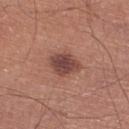| field | value |
|---|---|
| biopsy status | no biopsy performed (imaged during a skin exam) |
| image source | total-body-photography crop, ~15 mm field of view |
| lesion diameter | ~4 mm (longest diameter) |
| subject | male, aged 68–72 |
| body site | the right lower leg |